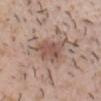Case summary:
* follow-up · catalogued during a skin exam; not biopsied
* subject · male, aged 23 to 27
* diameter · ~4.5 mm (longest diameter)
* lighting · white-light illumination
* anatomic site · the head or neck
* automated lesion analysis · lesion-presence confidence of about 100/100
* imaging modality · ~15 mm tile from a whole-body skin photo